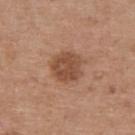workup: imaged on a skin check; not biopsied | automated metrics: a mean CIELAB color near L≈49 a*≈22 b*≈31 and a lesion-to-skin contrast of about 7.5 (normalized; higher = more distinct); an automated nevus-likeness rating near 50 out of 100 and a detector confidence of about 100 out of 100 that the crop contains a lesion | illumination: white-light illumination | image source: ~15 mm crop, total-body skin-cancer survey | anatomic site: the upper back | diameter: ≈4 mm | subject: female, about 40 years old.